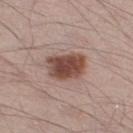Captured during whole-body skin photography for melanoma surveillance; the lesion was not biopsied.
The lesion is located on the right thigh.
A male patient, about 40 years old.
This is a white-light tile.
The recorded lesion diameter is about 4.5 mm.
A 15 mm close-up extracted from a 3D total-body photography capture.